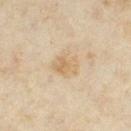Impression: Captured during whole-body skin photography for melanoma surveillance; the lesion was not biopsied. Background: A female subject, in their mid-30s. The lesion's longest dimension is about 3 mm. Cropped from a whole-body photographic skin survey; the tile spans about 15 mm. The lesion is on the right thigh.